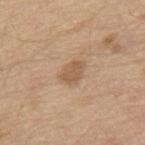Case summary:
* biopsy status — catalogued during a skin exam; not biopsied